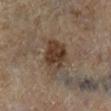Recorded during total-body skin imaging; not selected for excision or biopsy.
A lesion tile, about 15 mm wide, cut from a 3D total-body photograph.
The patient is a male aged around 85 or older.
From the right lower leg.